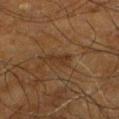This lesion was catalogued during total-body skin photography and was not selected for biopsy.
This is a cross-polarized tile.
The lesion is located on the left lower leg.
A lesion tile, about 15 mm wide, cut from a 3D total-body photograph.
Measured at roughly 3 mm in maximum diameter.
A male subject roughly 65 years of age.